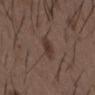About 3 mm across. On the chest. The patient is a male aged 48 to 52. A region of skin cropped from a whole-body photographic capture, roughly 15 mm wide. This is a white-light tile.